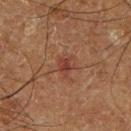Notes:
* biopsy status · catalogued during a skin exam; not biopsied
* body site · the right lower leg
* lesion diameter · about 2.5 mm
* tile lighting · cross-polarized illumination
* image · total-body-photography crop, ~15 mm field of view
* subject · male, aged 63–67